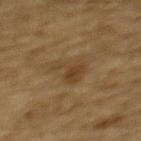This lesion was catalogued during total-body skin photography and was not selected for biopsy. A region of skin cropped from a whole-body photographic capture, roughly 15 mm wide. The patient is a male in their mid-80s or older. On the upper back.